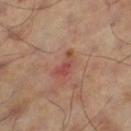| feature | finding |
|---|---|
| biopsy status | no biopsy performed (imaged during a skin exam) |
| anatomic site | the left thigh |
| lighting | cross-polarized |
| subject | male, about 60 years old |
| acquisition | ~15 mm crop, total-body skin-cancer survey |
| lesion size | ~3.5 mm (longest diameter) |